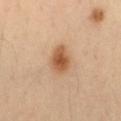<case>
<biopsy_status>not biopsied; imaged during a skin examination</biopsy_status>
<patient>
  <sex>male</sex>
  <age_approx>40</age_approx>
</patient>
<site>right thigh</site>
<lesion_size>
  <long_diameter_mm_approx>3.5</long_diameter_mm_approx>
</lesion_size>
<lighting>cross-polarized</lighting>
<automated_metrics>
  <border_irregularity_0_10>2.0</border_irregularity_0_10>
  <nevus_likeness_0_100>100</nevus_likeness_0_100>
  <lesion_detection_confidence_0_100>100</lesion_detection_confidence_0_100>
</automated_metrics>
<image>
  <source>total-body photography crop</source>
  <field_of_view_mm>15</field_of_view_mm>
</image>
</case>